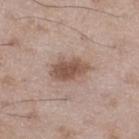An algorithmic analysis of the crop reported a mean CIELAB color near L≈52 a*≈17 b*≈26, a lesion–skin lightness drop of about 12, and a lesion-to-skin contrast of about 8.5 (normalized; higher = more distinct). And it measured a nevus-likeness score of about 80/100 and lesion-presence confidence of about 100/100. Measured at roughly 4.5 mm in maximum diameter. A male patient, about 50 years old. The lesion is located on the left thigh. A lesion tile, about 15 mm wide, cut from a 3D total-body photograph.